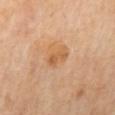  biopsy_status: not biopsied; imaged during a skin examination
  site: mid back
  lighting: cross-polarized
  image:
    source: total-body photography crop
    field_of_view_mm: 15
  automated_metrics:
    nevus_likeness_0_100: 5
    lesion_detection_confidence_0_100: 100